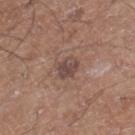This lesion was catalogued during total-body skin photography and was not selected for biopsy. A male subject in their 50s. Longest diameter approximately 3 mm. This image is a 15 mm lesion crop taken from a total-body photograph. On the right lower leg.